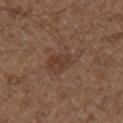<case>
  <biopsy_status>not biopsied; imaged during a skin examination</biopsy_status>
  <image>
    <source>total-body photography crop</source>
    <field_of_view_mm>15</field_of_view_mm>
  </image>
  <lesion_size>
    <long_diameter_mm_approx>5.0</long_diameter_mm_approx>
  </lesion_size>
  <site>right upper arm</site>
  <lighting>white-light</lighting>
  <patient>
    <sex>male</sex>
    <age_approx>50</age_approx>
  </patient>
</case>